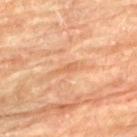Imaged during a routine full-body skin examination; the lesion was not biopsied and no histopathology is available. Located on the upper back. This image is a 15 mm lesion crop taken from a total-body photograph. The subject is a female aged 78–82.